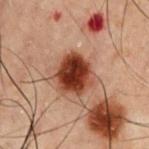From the chest. Cropped from a total-body skin-imaging series; the visible field is about 15 mm. Captured under cross-polarized illumination. A male subject aged approximately 60.